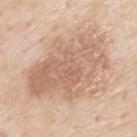- workup: imaged on a skin check; not biopsied
- image source: ~15 mm tile from a whole-body skin photo
- body site: the upper back
- subject: male, aged 78–82